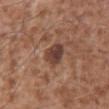Case summary:
* biopsy status — total-body-photography surveillance lesion; no biopsy
* anatomic site — the abdomen
* subject — male, approximately 45 years of age
* lighting — white-light
* acquisition — ~15 mm crop, total-body skin-cancer survey
* automated lesion analysis — a footprint of about 6 mm², an outline eccentricity of about 0.55 (0 = round, 1 = elongated), and a shape-asymmetry score of about 0.25 (0 = symmetric); a mean CIELAB color near L≈40 a*≈20 b*≈24, roughly 12 lightness units darker than nearby skin, and a normalized border contrast of about 10